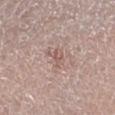Captured during whole-body skin photography for melanoma surveillance; the lesion was not biopsied.
Cropped from a total-body skin-imaging series; the visible field is about 15 mm.
The tile uses white-light illumination.
A female patient, aged 63 to 67.
The lesion is located on the right forearm.